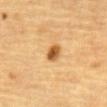This lesion was catalogued during total-body skin photography and was not selected for biopsy. The tile uses cross-polarized illumination. The subject is a male in their mid-80s or older. Cropped from a total-body skin-imaging series; the visible field is about 15 mm. Approximately 2.5 mm at its widest. The lesion is on the abdomen.